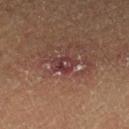The lesion was tiled from a total-body skin photograph and was not biopsied. An algorithmic analysis of the crop reported a lesion color around L≈31 a*≈21 b*≈17 in CIELAB, roughly 7 lightness units darker than nearby skin, and a lesion-to-skin contrast of about 7.5 (normalized; higher = more distinct). The analysis additionally found a border-irregularity rating of about 2.5/10. The software also gave an automated nevus-likeness rating near 0 out of 100 and a lesion-detection confidence of about 90/100. A roughly 15 mm field-of-view crop from a total-body skin photograph. Longest diameter approximately 2.5 mm. A male patient aged 58 to 62.